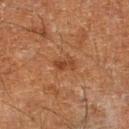<record>
<biopsy_status>not biopsied; imaged during a skin examination</biopsy_status>
<patient>
  <sex>male</sex>
  <age_approx>60</age_approx>
</patient>
<image>
  <source>total-body photography crop</source>
  <field_of_view_mm>15</field_of_view_mm>
</image>
<lesion_size>
  <long_diameter_mm_approx>2.5</long_diameter_mm_approx>
</lesion_size>
<site>right lower leg</site>
</record>